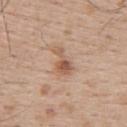Impression: Captured during whole-body skin photography for melanoma surveillance; the lesion was not biopsied. Background: The patient is a male aged 63–67. A 15 mm close-up extracted from a 3D total-body photography capture. On the back.